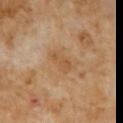Assessment: This lesion was catalogued during total-body skin photography and was not selected for biopsy. Image and clinical context: On the abdomen. Automated image analysis of the tile measured a lesion-to-skin contrast of about 5.5 (normalized; higher = more distinct). A 15 mm crop from a total-body photograph taken for skin-cancer surveillance. A male patient approximately 60 years of age. The recorded lesion diameter is about 3 mm. Imaged with cross-polarized lighting.